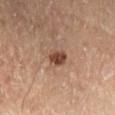Clinical impression:
Part of a total-body skin-imaging series; this lesion was reviewed on a skin check and was not flagged for biopsy.
Acquisition and patient details:
The lesion is on the left lower leg. A male patient, roughly 65 years of age. Approximately 2.5 mm at its widest. Automated tile analysis of the lesion measured a mean CIELAB color near L≈44 a*≈21 b*≈28, a lesion–skin lightness drop of about 13, and a normalized lesion–skin contrast near 10. It also reported a border-irregularity index near 2/10, internal color variation of about 3.5 on a 0–10 scale, and radial color variation of about 1.5. A 15 mm close-up extracted from a 3D total-body photography capture.